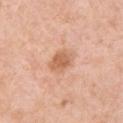Case summary:
• follow-up: no biopsy performed (imaged during a skin exam)
• lesion diameter: about 3 mm
• illumination: white-light illumination
• patient: female, roughly 70 years of age
• imaging modality: ~15 mm crop, total-body skin-cancer survey
• body site: the right upper arm
• automated lesion analysis: a lesion color around L≈63 a*≈23 b*≈34 in CIELAB, roughly 10 lightness units darker than nearby skin, and a normalized lesion–skin contrast near 7; a border-irregularity index near 2/10 and internal color variation of about 2 on a 0–10 scale